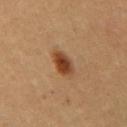Recorded during total-body skin imaging; not selected for excision or biopsy. From the mid back. Imaged with cross-polarized lighting. The lesion's longest dimension is about 3 mm. A male patient, in their mid-50s. Cropped from a whole-body photographic skin survey; the tile spans about 15 mm.